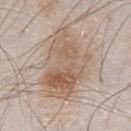The lesion was photographed on a routine skin check and not biopsied; there is no pathology result. Longest diameter approximately 8 mm. Located on the front of the torso. The lesion-visualizer software estimated a lesion area of about 29 mm², an eccentricity of roughly 0.8, and a symmetry-axis asymmetry near 0.35. The software also gave a classifier nevus-likeness of about 55/100 and lesion-presence confidence of about 100/100. The tile uses white-light illumination. The patient is a male about 80 years old. A 15 mm close-up extracted from a 3D total-body photography capture.